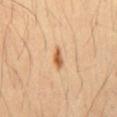{
  "biopsy_status": "not biopsied; imaged during a skin examination",
  "image": {
    "source": "total-body photography crop",
    "field_of_view_mm": 15
  },
  "site": "mid back",
  "lighting": "cross-polarized",
  "lesion_size": {
    "long_diameter_mm_approx": 3.0
  },
  "patient": {
    "sex": "male",
    "age_approx": 50
  }
}